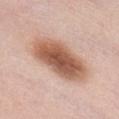The lesion was tiled from a total-body skin photograph and was not biopsied.
The lesion is located on the abdomen.
Cropped from a whole-body photographic skin survey; the tile spans about 15 mm.
This is a white-light tile.
The subject is a female aged around 50.
The recorded lesion diameter is about 9 mm.